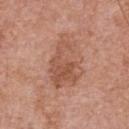The lesion was tiled from a total-body skin photograph and was not biopsied. Cropped from a whole-body photographic skin survey; the tile spans about 15 mm. Located on the chest. The tile uses white-light illumination. Longest diameter approximately 6 mm. The lesion-visualizer software estimated a mean CIELAB color near L≈54 a*≈23 b*≈30 and a normalized lesion–skin contrast near 6.5. It also reported a border-irregularity index near 6.5/10, a color-variation rating of about 4.5/10, and a peripheral color-asymmetry measure near 1.5. A male patient, aged 68 to 72.